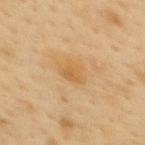| key | value |
|---|---|
| follow-up | no biopsy performed (imaged during a skin exam) |
| location | the back |
| lighting | cross-polarized |
| image source | 15 mm crop, total-body photography |
| diameter | ≈2.5 mm |
| patient | female, about 50 years old |